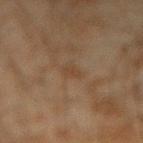biopsy_status: not biopsied; imaged during a skin examination
lesion_size:
  long_diameter_mm_approx: 2.5
image:
  source: total-body photography crop
  field_of_view_mm: 15
lighting: cross-polarized
patient:
  sex: male
  age_approx: 45
site: left forearm
automated_metrics:
  cielab_L: 34
  cielab_a: 13
  cielab_b: 25
  vs_skin_contrast_norm: 4.5
  border_irregularity_0_10: 4.0
  color_variation_0_10: 1.0
  peripheral_color_asymmetry: 0.5
  nevus_likeness_0_100: 0
  lesion_detection_confidence_0_100: 100